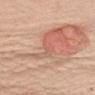Assessment: No biopsy was performed on this lesion — it was imaged during a full skin examination and was not determined to be concerning. Background: A female patient, roughly 45 years of age. The total-body-photography lesion software estimated a footprint of about 60 mm², a shape eccentricity near 0.85, and two-axis asymmetry of about 0.65. It also reported a lesion color around L≈64 a*≈21 b*≈30 in CIELAB. The lesion's longest dimension is about 16 mm. On the left upper arm. A 15 mm crop from a total-body photograph taken for skin-cancer surveillance.